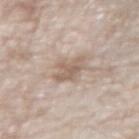Clinical impression:
The lesion was photographed on a routine skin check and not biopsied; there is no pathology result.
Clinical summary:
Automated tile analysis of the lesion measured a footprint of about 6.5 mm², an eccentricity of roughly 0.75, and two-axis asymmetry of about 0.3. It also reported an average lesion color of about L≈60 a*≈14 b*≈25 (CIELAB), roughly 9 lightness units darker than nearby skin, and a normalized lesion–skin contrast near 6.5. The software also gave border irregularity of about 4 on a 0–10 scale, a within-lesion color-variation index near 2/10, and peripheral color asymmetry of about 1. And it measured a nevus-likeness score of about 0/100. A 15 mm crop from a total-body photograph taken for skin-cancer surveillance. The lesion is on the right forearm. A female patient aged 73–77. Longest diameter approximately 3.5 mm.